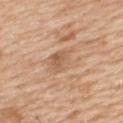Clinical impression: The lesion was photographed on a routine skin check and not biopsied; there is no pathology result. Context: A roughly 15 mm field-of-view crop from a total-body skin photograph. A male subject, aged 58–62. On the upper back.